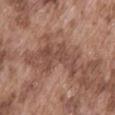Q: Was a biopsy performed?
A: imaged on a skin check; not biopsied
Q: How was the tile lit?
A: white-light illumination
Q: Patient demographics?
A: male, aged approximately 75
Q: Lesion size?
A: ~5.5 mm (longest diameter)
Q: What did automated image analysis measure?
A: an area of roughly 12 mm², an outline eccentricity of about 0.8 (0 = round, 1 = elongated), and two-axis asymmetry of about 0.6; an average lesion color of about L≈47 a*≈20 b*≈26 (CIELAB) and a normalized border contrast of about 6; a border-irregularity rating of about 8.5/10, internal color variation of about 3 on a 0–10 scale, and a peripheral color-asymmetry measure near 1
Q: Where on the body is the lesion?
A: the abdomen
Q: What kind of image is this?
A: 15 mm crop, total-body photography